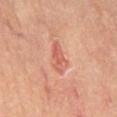- subject · male, in their 70s
- anatomic site · the mid back
- size · ~4 mm (longest diameter)
- tile lighting · cross-polarized illumination
- acquisition · 15 mm crop, total-body photography